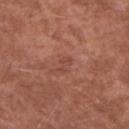Q: Was this lesion biopsied?
A: catalogued during a skin exam; not biopsied
Q: Patient demographics?
A: male, aged approximately 55
Q: What kind of image is this?
A: total-body-photography crop, ~15 mm field of view
Q: Lesion location?
A: the left upper arm
Q: What is the lesion's diameter?
A: about 2.5 mm